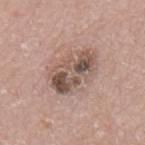Captured during whole-body skin photography for melanoma surveillance; the lesion was not biopsied.
From the mid back.
A roughly 15 mm field-of-view crop from a total-body skin photograph.
A male patient, roughly 70 years of age.